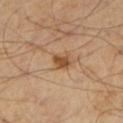| field | value |
|---|---|
| follow-up | imaged on a skin check; not biopsied |
| illumination | cross-polarized |
| size | about 2 mm |
| body site | the left lower leg |
| acquisition | 15 mm crop, total-body photography |
| patient | male, in their mid-50s |
| automated lesion analysis | a lesion color around L≈47 a*≈20 b*≈36 in CIELAB, about 12 CIELAB-L* units darker than the surrounding skin, and a lesion-to-skin contrast of about 9.5 (normalized; higher = more distinct); an automated nevus-likeness rating near 85 out of 100 |